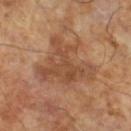{"biopsy_status": "not biopsied; imaged during a skin examination", "lighting": "cross-polarized", "patient": {"sex": "male", "age_approx": 65}, "automated_metrics": {"area_mm2_approx": 24.0, "nevus_likeness_0_100": 5, "lesion_detection_confidence_0_100": 100}, "lesion_size": {"long_diameter_mm_approx": 7.0}, "image": {"source": "total-body photography crop", "field_of_view_mm": 15}}